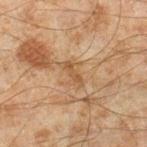<tbp_lesion>
  <biopsy_status>not biopsied; imaged during a skin examination</biopsy_status>
  <image>
    <source>total-body photography crop</source>
    <field_of_view_mm>15</field_of_view_mm>
  </image>
  <patient>
    <sex>male</sex>
    <age_approx>45</age_approx>
  </patient>
  <automated_metrics>
    <area_mm2_approx>3.0</area_mm2_approx>
    <eccentricity>0.95</eccentricity>
    <cielab_L>43</cielab_L>
    <cielab_a>16</cielab_a>
    <cielab_b>31</cielab_b>
    <vs_skin_contrast_norm>6.5</vs_skin_contrast_norm>
    <color_variation_0_10>0.0</color_variation_0_10>
    <peripheral_color_asymmetry>0.0</peripheral_color_asymmetry>
  </automated_metrics>
  <site>right lower leg</site>
  <lesion_size>
    <long_diameter_mm_approx>3.0</long_diameter_mm_approx>
  </lesion_size>
</tbp_lesion>